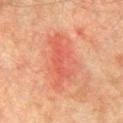• acquisition — total-body-photography crop, ~15 mm field of view
• location — the chest
• patient — male, in their mid-70s
• automated lesion analysis — a lesion color around L≈50 a*≈28 b*≈30 in CIELAB and roughly 7 lightness units darker than nearby skin
• tile lighting — cross-polarized
• size — ~7.5 mm (longest diameter)